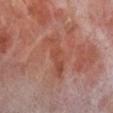Notes:
* notes — imaged on a skin check; not biopsied
* site — the right lower leg
* imaging modality — total-body-photography crop, ~15 mm field of view
* tile lighting — cross-polarized illumination
* size — ~5.5 mm (longest diameter)
* subject — male, approximately 70 years of age